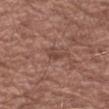subject: male, in their mid-60s
anatomic site: the left forearm
size: about 2.5 mm
image source: ~15 mm crop, total-body skin-cancer survey
TBP lesion metrics: an automated nevus-likeness rating near 0 out of 100